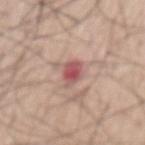biopsy status = catalogued during a skin exam; not biopsied
subject = male, aged 58–62
site = the mid back
acquisition = 15 mm crop, total-body photography
lesion diameter = about 3.5 mm
tile lighting = white-light
TBP lesion metrics = a mean CIELAB color near L≈55 a*≈27 b*≈22 and about 12 CIELAB-L* units darker than the surrounding skin; lesion-presence confidence of about 100/100